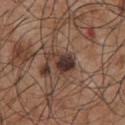Case summary:
* biopsy status — catalogued during a skin exam; not biopsied
* lesion size — ~4 mm (longest diameter)
* tile lighting — white-light
* location — the chest
* acquisition — 15 mm crop, total-body photography
* patient — male, in their mid-50s